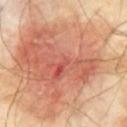Assessment: Captured during whole-body skin photography for melanoma surveillance; the lesion was not biopsied. Acquisition and patient details: The patient is a male roughly 70 years of age. A 15 mm close-up tile from a total-body photography series done for melanoma screening. The lesion is located on the front of the torso.